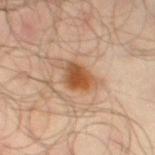| key | value |
|---|---|
| image source | ~15 mm crop, total-body skin-cancer survey |
| patient | male, in their mid- to late 60s |
| tile lighting | cross-polarized |
| body site | the left thigh |
| automated metrics | a footprint of about 7 mm², an outline eccentricity of about 0.7 (0 = round, 1 = elongated), and a shape-asymmetry score of about 0.3 (0 = symmetric); a lesion-detection confidence of about 100/100 |